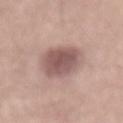The lesion was photographed on a routine skin check and not biopsied; there is no pathology result. A male subject, aged 53 to 57. An algorithmic analysis of the crop reported an area of roughly 15 mm² and a symmetry-axis asymmetry near 0.15. The analysis additionally found border irregularity of about 1.5 on a 0–10 scale, internal color variation of about 3 on a 0–10 scale, and peripheral color asymmetry of about 1. The analysis additionally found a nevus-likeness score of about 45/100 and a lesion-detection confidence of about 100/100. Longest diameter approximately 5.5 mm. A roughly 15 mm field-of-view crop from a total-body skin photograph. The lesion is on the back. Captured under white-light illumination.